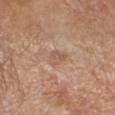Recorded during total-body skin imaging; not selected for excision or biopsy. Longest diameter approximately 3 mm. The total-body-photography lesion software estimated a mean CIELAB color near L≈56 a*≈18 b*≈29 and a lesion-to-skin contrast of about 5 (normalized; higher = more distinct). It also reported a border-irregularity index near 3/10, a within-lesion color-variation index near 2/10, and a peripheral color-asymmetry measure near 1. The software also gave an automated nevus-likeness rating near 0 out of 100 and a lesion-detection confidence of about 100/100. The lesion is on the arm. A region of skin cropped from a whole-body photographic capture, roughly 15 mm wide. The subject is a female aged around 55. Imaged with cross-polarized lighting.